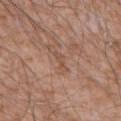This lesion was catalogued during total-body skin photography and was not selected for biopsy.
Automated image analysis of the tile measured a mean CIELAB color near L≈51 a*≈20 b*≈29 and a lesion-to-skin contrast of about 5 (normalized; higher = more distinct). And it measured an automated nevus-likeness rating near 0 out of 100 and a detector confidence of about 75 out of 100 that the crop contains a lesion.
A close-up tile cropped from a whole-body skin photograph, about 15 mm across.
The lesion's longest dimension is about 3 mm.
Imaged with white-light lighting.
A male subject aged 58–62.
The lesion is located on the mid back.